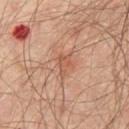biopsy_status: not biopsied; imaged during a skin examination
image:
  source: total-body photography crop
  field_of_view_mm: 15
site: chest
patient:
  sex: male
  age_approx: 65
lighting: cross-polarized
lesion_size:
  long_diameter_mm_approx: 3.0